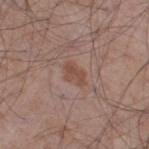Captured during whole-body skin photography for melanoma surveillance; the lesion was not biopsied. A male subject aged 53 to 57. Measured at roughly 3 mm in maximum diameter. The lesion is on the right thigh. A 15 mm crop from a total-body photograph taken for skin-cancer surveillance. Automated image analysis of the tile measured a lesion area of about 4 mm², a shape eccentricity near 0.8, and a shape-asymmetry score of about 0.4 (0 = symmetric). It also reported a mean CIELAB color near L≈48 a*≈20 b*≈26, about 7 CIELAB-L* units darker than the surrounding skin, and a normalized lesion–skin contrast near 6.5. The analysis additionally found a border-irregularity rating of about 4/10, internal color variation of about 1 on a 0–10 scale, and a peripheral color-asymmetry measure near 0.5. And it measured a nevus-likeness score of about 15/100 and a detector confidence of about 100 out of 100 that the crop contains a lesion. This is a white-light tile.